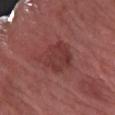| feature | finding |
|---|---|
| notes | total-body-photography surveillance lesion; no biopsy |
| subject | female, about 60 years old |
| image source | ~15 mm crop, total-body skin-cancer survey |
| body site | the right forearm |
| diameter | about 5.5 mm |
| lighting | white-light illumination |